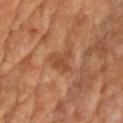Q: Is there a histopathology result?
A: total-body-photography surveillance lesion; no biopsy
Q: What is the anatomic site?
A: the chest
Q: What is the lesion's diameter?
A: ~3.5 mm (longest diameter)
Q: How was the tile lit?
A: cross-polarized
Q: What is the imaging modality?
A: 15 mm crop, total-body photography
Q: Automated lesion metrics?
A: a lesion–skin lightness drop of about 8 and a normalized lesion–skin contrast near 6
Q: Patient demographics?
A: male, aged around 65